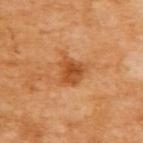Q: Is there a histopathology result?
A: no biopsy performed (imaged during a skin exam)
Q: What is the lesion's diameter?
A: ≈3.5 mm
Q: What did automated image analysis measure?
A: a symmetry-axis asymmetry near 0.3; a mean CIELAB color near L≈53 a*≈28 b*≈44 and roughly 11 lightness units darker than nearby skin
Q: What are the patient's age and sex?
A: female, in their mid- to late 50s
Q: How was the tile lit?
A: cross-polarized
Q: What kind of image is this?
A: ~15 mm crop, total-body skin-cancer survey
Q: Where on the body is the lesion?
A: the upper back